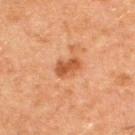biopsy status: imaged on a skin check; not biopsied
illumination: cross-polarized illumination
TBP lesion metrics: border irregularity of about 2.5 on a 0–10 scale, internal color variation of about 2.5 on a 0–10 scale, and radial color variation of about 1; a nevus-likeness score of about 75/100 and a lesion-detection confidence of about 100/100
subject: male, aged 48 to 52
image: ~15 mm crop, total-body skin-cancer survey
location: the upper back